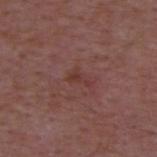Recorded during total-body skin imaging; not selected for excision or biopsy.
A male subject, aged 48 to 52.
The total-body-photography lesion software estimated an outline eccentricity of about 0.85 (0 = round, 1 = elongated) and a shape-asymmetry score of about 0.6 (0 = symmetric). The analysis additionally found border irregularity of about 6 on a 0–10 scale, a within-lesion color-variation index near 0/10, and radial color variation of about 0.
Captured under white-light illumination.
Measured at roughly 2.5 mm in maximum diameter.
A lesion tile, about 15 mm wide, cut from a 3D total-body photograph.
The lesion is on the upper back.